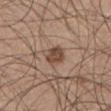Assessment: Imaged during a routine full-body skin examination; the lesion was not biopsied and no histopathology is available. Acquisition and patient details: This is a white-light tile. About 3 mm across. The lesion is on the abdomen. A male subject, aged 73 to 77. The lesion-visualizer software estimated border irregularity of about 2 on a 0–10 scale, a color-variation rating of about 2.5/10, and peripheral color asymmetry of about 1. The analysis additionally found an automated nevus-likeness rating near 70 out of 100 and a detector confidence of about 100 out of 100 that the crop contains a lesion. A 15 mm close-up tile from a total-body photography series done for melanoma screening.